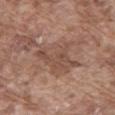The lesion was tiled from a total-body skin photograph and was not biopsied.
A male patient, approximately 75 years of age.
Imaged with white-light lighting.
A lesion tile, about 15 mm wide, cut from a 3D total-body photograph.
The lesion is located on the mid back.